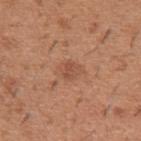| field | value |
|---|---|
| follow-up | no biopsy performed (imaged during a skin exam) |
| location | the left upper arm |
| imaging modality | total-body-photography crop, ~15 mm field of view |
| patient | male, in their 40s |
| lesion diameter | ≈2.5 mm |
| TBP lesion metrics | a border-irregularity rating of about 2/10, a color-variation rating of about 2/10, and a peripheral color-asymmetry measure near 1 |
| tile lighting | white-light illumination |